A close-up tile cropped from a whole-body skin photograph, about 15 mm across. A female patient, in their 40s. On the upper back.
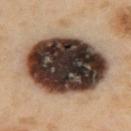On biopsy, histopathology showed a seborrheic keratosis.